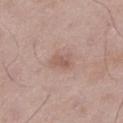No biopsy was performed on this lesion — it was imaged during a full skin examination and was not determined to be concerning. Cropped from a whole-body photographic skin survey; the tile spans about 15 mm. On the left thigh. A male patient aged approximately 50.